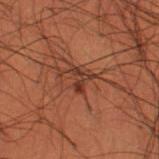Assessment: This lesion was catalogued during total-body skin photography and was not selected for biopsy. Image and clinical context: A close-up tile cropped from a whole-body skin photograph, about 15 mm across. This is a cross-polarized tile. Approximately 3 mm at its widest. The total-body-photography lesion software estimated a footprint of about 3 mm², a shape eccentricity near 0.85, and two-axis asymmetry of about 0.55. It also reported a border-irregularity index near 6/10, internal color variation of about 0 on a 0–10 scale, and a peripheral color-asymmetry measure near 0. It also reported a nevus-likeness score of about 0/100. The lesion is located on the leg. A male patient aged 48–52.